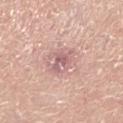Impression: The lesion was tiled from a total-body skin photograph and was not biopsied. Acquisition and patient details: Measured at roughly 4 mm in maximum diameter. The patient is a female aged approximately 65. Imaged with white-light lighting. Cropped from a total-body skin-imaging series; the visible field is about 15 mm. Automated tile analysis of the lesion measured a lesion–skin lightness drop of about 9 and a normalized border contrast of about 6. It also reported a within-lesion color-variation index near 5.5/10 and peripheral color asymmetry of about 2. It also reported an automated nevus-likeness rating near 0 out of 100 and a lesion-detection confidence of about 100/100. Located on the left lower leg.